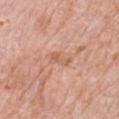  biopsy_status: not biopsied; imaged during a skin examination
  lesion_size:
    long_diameter_mm_approx: 3.0
  site: chest
  patient:
    sex: male
    age_approx: 80
  lighting: white-light
  image:
    source: total-body photography crop
    field_of_view_mm: 15